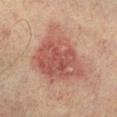Q: Is there a histopathology result?
A: catalogued during a skin exam; not biopsied
Q: Automated lesion metrics?
A: a lesion color around L≈45 a*≈22 b*≈23 in CIELAB, about 10 CIELAB-L* units darker than the surrounding skin, and a normalized lesion–skin contrast near 7.5
Q: Illumination type?
A: cross-polarized illumination
Q: Where on the body is the lesion?
A: the right lower leg
Q: What is the imaging modality?
A: 15 mm crop, total-body photography
Q: What are the patient's age and sex?
A: male, aged around 75
Q: What is the lesion's diameter?
A: ≈8 mm